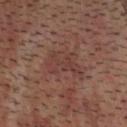follow-up: total-body-photography surveillance lesion; no biopsy
subject: male, aged 58–62
size: about 4.5 mm
tile lighting: cross-polarized illumination
site: the head or neck
image: ~15 mm crop, total-body skin-cancer survey